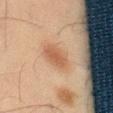Findings:
- follow-up · imaged on a skin check; not biopsied
- site · the leg
- acquisition · ~15 mm crop, total-body skin-cancer survey
- tile lighting · cross-polarized
- patient · male, approximately 45 years of age
- lesion diameter · about 4.5 mm